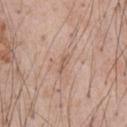Clinical impression: The lesion was photographed on a routine skin check and not biopsied; there is no pathology result. Clinical summary: The recorded lesion diameter is about 2.5 mm. A male subject, about 55 years old. Imaged with white-light lighting. A roughly 15 mm field-of-view crop from a total-body skin photograph. On the chest.